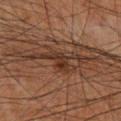The lesion was tiled from a total-body skin photograph and was not biopsied. Approximately 7 mm at its widest. A male patient aged 58–62. Located on the back. A 15 mm close-up tile from a total-body photography series done for melanoma screening.